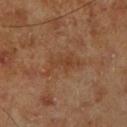Notes:
* follow-up · total-body-photography surveillance lesion; no biopsy
* image · ~15 mm crop, total-body skin-cancer survey
* subject · male, about 70 years old
* body site · the leg